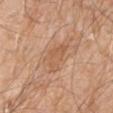<lesion>
  <biopsy_status>not biopsied; imaged during a skin examination</biopsy_status>
  <site>right upper arm</site>
  <lighting>white-light</lighting>
  <lesion_size>
    <long_diameter_mm_approx>4.0</long_diameter_mm_approx>
  </lesion_size>
  <image>
    <source>total-body photography crop</source>
    <field_of_view_mm>15</field_of_view_mm>
  </image>
  <patient>
    <sex>male</sex>
    <age_approx>80</age_approx>
  </patient>
</lesion>